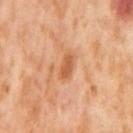<case>
  <biopsy_status>not biopsied; imaged during a skin examination</biopsy_status>
  <image>
    <source>total-body photography crop</source>
    <field_of_view_mm>15</field_of_view_mm>
  </image>
  <site>left thigh</site>
  <automated_metrics>
    <area_mm2_approx>4.0</area_mm2_approx>
    <eccentricity>0.9</eccentricity>
    <shape_asymmetry>0.35</shape_asymmetry>
    <cielab_L>60</cielab_L>
    <cielab_a>28</cielab_a>
    <cielab_b>40</cielab_b>
    <vs_skin_darker_L>11.0</vs_skin_darker_L>
    <vs_skin_contrast_norm>7.0</vs_skin_contrast_norm>
    <border_irregularity_0_10>3.5</border_irregularity_0_10>
    <peripheral_color_asymmetry>0.5</peripheral_color_asymmetry>
  </automated_metrics>
  <lighting>cross-polarized</lighting>
  <patient>
    <sex>female</sex>
    <age_approx>55</age_approx>
  </patient>
</case>